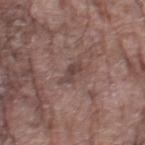Clinical impression:
Imaged during a routine full-body skin examination; the lesion was not biopsied and no histopathology is available.
Image and clinical context:
A male patient aged around 80. A lesion tile, about 15 mm wide, cut from a 3D total-body photograph. On the leg.